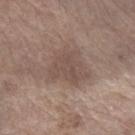Assessment: Captured during whole-body skin photography for melanoma surveillance; the lesion was not biopsied. Image and clinical context: On the right forearm. A male patient aged around 70. This is a white-light tile. Approximately 4.5 mm at its widest. Cropped from a total-body skin-imaging series; the visible field is about 15 mm.